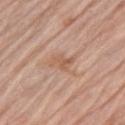Imaged during a routine full-body skin examination; the lesion was not biopsied and no histopathology is available.
Captured under white-light illumination.
A 15 mm close-up tile from a total-body photography series done for melanoma screening.
A male patient, aged 78 to 82.
Located on the left upper arm.
The recorded lesion diameter is about 3 mm.